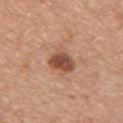Captured during whole-body skin photography for melanoma surveillance; the lesion was not biopsied. Located on the front of the torso. An algorithmic analysis of the crop reported a lesion color around L≈51 a*≈22 b*≈31 in CIELAB, a lesion–skin lightness drop of about 13, and a normalized lesion–skin contrast near 9. And it measured an automated nevus-likeness rating near 75 out of 100 and lesion-presence confidence of about 100/100. A male patient aged 58 to 62. Longest diameter approximately 3.5 mm. The tile uses white-light illumination. A region of skin cropped from a whole-body photographic capture, roughly 15 mm wide.